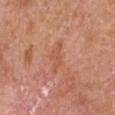<record>
<biopsy_status>not biopsied; imaged during a skin examination</biopsy_status>
<lighting>white-light</lighting>
<automated_metrics>
  <area_mm2_approx>5.0</area_mm2_approx>
  <shape_asymmetry>0.4</shape_asymmetry>
  <border_irregularity_0_10>5.5</border_irregularity_0_10>
  <peripheral_color_asymmetry>0.5</peripheral_color_asymmetry>
  <nevus_likeness_0_100>0</nevus_likeness_0_100>
</automated_metrics>
<patient>
  <sex>male</sex>
  <age_approx>65</age_approx>
</patient>
<image>
  <source>total-body photography crop</source>
  <field_of_view_mm>15</field_of_view_mm>
</image>
<site>chest</site>
</record>